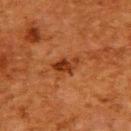follow-up = no biopsy performed (imaged during a skin exam)
image-analysis metrics = a shape eccentricity near 0.8 and two-axis asymmetry of about 0.35; a classifier nevus-likeness of about 75/100 and a detector confidence of about 100 out of 100 that the crop contains a lesion
patient = female, aged 48 to 52
image source = ~15 mm crop, total-body skin-cancer survey
anatomic site = the back
tile lighting = cross-polarized